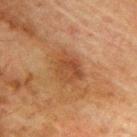A male subject, aged around 75. This image is a 15 mm lesion crop taken from a total-body photograph. The lesion is located on the upper back. Longest diameter approximately 3.5 mm.